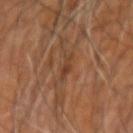Imaged during a routine full-body skin examination; the lesion was not biopsied and no histopathology is available. The lesion is on the right upper arm. A male patient aged 58 to 62. A lesion tile, about 15 mm wide, cut from a 3D total-body photograph.